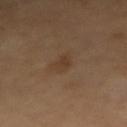Clinical impression: No biopsy was performed on this lesion — it was imaged during a full skin examination and was not determined to be concerning. Clinical summary: From the arm. This image is a 15 mm lesion crop taken from a total-body photograph. The patient is roughly 60 years of age. Longest diameter approximately 2.5 mm. Captured under cross-polarized illumination. An algorithmic analysis of the crop reported a border-irregularity rating of about 2.5/10 and a peripheral color-asymmetry measure near 0.5.